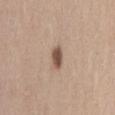Notes:
- biopsy status · no biopsy performed (imaged during a skin exam)
- lesion diameter · ≈2.5 mm
- subject · female, aged 38 to 42
- tile lighting · white-light illumination
- site · the lower back
- TBP lesion metrics · a shape eccentricity near 0.7 and a shape-asymmetry score of about 0.2 (0 = symmetric); an average lesion color of about L≈52 a*≈17 b*≈26 (CIELAB), a lesion–skin lightness drop of about 15, and a lesion-to-skin contrast of about 10 (normalized; higher = more distinct)
- acquisition · ~15 mm crop, total-body skin-cancer survey